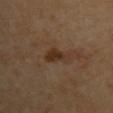{"biopsy_status": "not biopsied; imaged during a skin examination", "image": {"source": "total-body photography crop", "field_of_view_mm": 15}, "patient": {"sex": "female", "age_approx": 70}, "automated_metrics": {"cielab_L": 32, "cielab_a": 17, "cielab_b": 28, "vs_skin_contrast_norm": 8.0}, "site": "upper back", "lighting": "cross-polarized", "lesion_size": {"long_diameter_mm_approx": 3.5}}